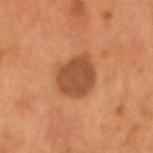Part of a total-body skin-imaging series; this lesion was reviewed on a skin check and was not flagged for biopsy.
A male subject aged 53–57.
Measured at roughly 4 mm in maximum diameter.
This is a cross-polarized tile.
Located on the head or neck.
Cropped from a whole-body photographic skin survey; the tile spans about 15 mm.